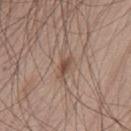– tile lighting: white-light illumination
– image source: ~15 mm tile from a whole-body skin photo
– anatomic site: the front of the torso
– subject: male, about 65 years old
– lesion size: about 3 mm